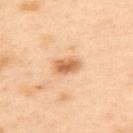| feature | finding |
|---|---|
| workup | total-body-photography surveillance lesion; no biopsy |
| image | ~15 mm crop, total-body skin-cancer survey |
| subject | female, in their 40s |
| lesion size | ~3 mm (longest diameter) |
| automated lesion analysis | an area of roughly 5 mm², an eccentricity of roughly 0.8, and a symmetry-axis asymmetry near 0.15; border irregularity of about 1.5 on a 0–10 scale, a within-lesion color-variation index near 4/10, and radial color variation of about 1.5 |
| location | the upper back |
| lighting | cross-polarized illumination |